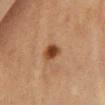Recorded during total-body skin imaging; not selected for excision or biopsy. A female patient, in their mid-60s. Located on the chest. The lesion's longest dimension is about 2.5 mm. A close-up tile cropped from a whole-body skin photograph, about 15 mm across. Captured under cross-polarized illumination. Automated tile analysis of the lesion measured a mean CIELAB color near L≈37 a*≈21 b*≈31 and roughly 13 lightness units darker than nearby skin.